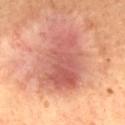Q: Was a biopsy performed?
A: imaged on a skin check; not biopsied
Q: Lesion size?
A: ~7.5 mm (longest diameter)
Q: What are the patient's age and sex?
A: male, aged 48 to 52
Q: How was this image acquired?
A: ~15 mm crop, total-body skin-cancer survey
Q: Lesion location?
A: the mid back
Q: Illumination type?
A: cross-polarized